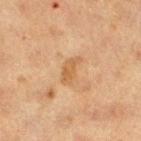Impression:
No biopsy was performed on this lesion — it was imaged during a full skin examination and was not determined to be concerning.
Clinical summary:
Longest diameter approximately 3 mm. The subject is a female aged around 40. Automated tile analysis of the lesion measured a footprint of about 4.5 mm² and an outline eccentricity of about 0.85 (0 = round, 1 = elongated). The analysis additionally found a detector confidence of about 100 out of 100 that the crop contains a lesion. A roughly 15 mm field-of-view crop from a total-body skin photograph. Imaged with cross-polarized lighting. On the right thigh.